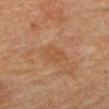Q: Is there a histopathology result?
A: no biopsy performed (imaged during a skin exam)
Q: What kind of image is this?
A: ~15 mm crop, total-body skin-cancer survey
Q: What are the patient's age and sex?
A: male, aged 78 to 82
Q: Lesion location?
A: the abdomen
Q: How large is the lesion?
A: about 3 mm
Q: Automated lesion metrics?
A: border irregularity of about 1.5 on a 0–10 scale, a color-variation rating of about 1.5/10, and a peripheral color-asymmetry measure near 0.5